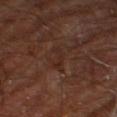A male subject roughly 60 years of age.
On the right leg.
A lesion tile, about 15 mm wide, cut from a 3D total-body photograph.
Captured under cross-polarized illumination.
The total-body-photography lesion software estimated a border-irregularity index near 6.5/10, a within-lesion color-variation index near 0/10, and a peripheral color-asymmetry measure near 0. The software also gave an automated nevus-likeness rating near 0 out of 100 and a detector confidence of about 60 out of 100 that the crop contains a lesion.
About 3 mm across.